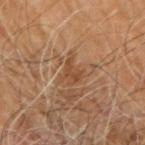Background:
A male subject in their 60s. About 3 mm across. A lesion tile, about 15 mm wide, cut from a 3D total-body photograph. The lesion is on the right arm. The tile uses cross-polarized illumination. The total-body-photography lesion software estimated a lesion area of about 4 mm², an eccentricity of roughly 0.6, and a symmetry-axis asymmetry near 0.45. The software also gave a lesion color around L≈45 a*≈21 b*≈33 in CIELAB and a lesion-to-skin contrast of about 6.5 (normalized; higher = more distinct).